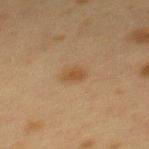Q: Was a biopsy performed?
A: no biopsy performed (imaged during a skin exam)
Q: What lighting was used for the tile?
A: cross-polarized illumination
Q: How was this image acquired?
A: 15 mm crop, total-body photography
Q: Automated lesion metrics?
A: a lesion area of about 3.5 mm², a shape eccentricity near 0.7, and a shape-asymmetry score of about 0.2 (0 = symmetric); a lesion–skin lightness drop of about 6 and a normalized border contrast of about 6.5
Q: Where on the body is the lesion?
A: the upper back
Q: How large is the lesion?
A: about 2.5 mm
Q: Patient demographics?
A: female, roughly 40 years of age